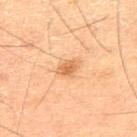notes: imaged on a skin check; not biopsied
patient: male, in their 60s
acquisition: total-body-photography crop, ~15 mm field of view
illumination: cross-polarized
lesion size: about 3 mm